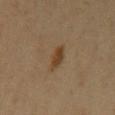Clinical impression: This lesion was catalogued during total-body skin photography and was not selected for biopsy. Context: The total-body-photography lesion software estimated a border-irregularity rating of about 2.5/10, internal color variation of about 1.5 on a 0–10 scale, and peripheral color asymmetry of about 0.5. On the left upper arm. A 15 mm close-up tile from a total-body photography series done for melanoma screening. Captured under cross-polarized illumination. A female patient, aged 43 to 47. Longest diameter approximately 2.5 mm.